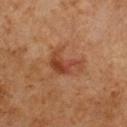Notes:
* notes: imaged on a skin check; not biopsied
* image: ~15 mm tile from a whole-body skin photo
* tile lighting: cross-polarized
* size: ~4 mm (longest diameter)
* TBP lesion metrics: an area of roughly 8 mm² and an outline eccentricity of about 0.75 (0 = round, 1 = elongated); a lesion color around L≈43 a*≈26 b*≈33 in CIELAB, a lesion–skin lightness drop of about 9, and a lesion-to-skin contrast of about 7.5 (normalized; higher = more distinct); border irregularity of about 7 on a 0–10 scale, a color-variation rating of about 4.5/10, and a peripheral color-asymmetry measure near 1.5
* patient: male, aged 63 to 67